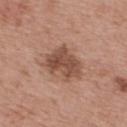Context: The lesion is on the upper back. The tile uses white-light illumination. The subject is a male aged 63–67. About 4.5 mm across. A region of skin cropped from a whole-body photographic capture, roughly 15 mm wide.